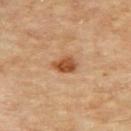Part of a total-body skin-imaging series; this lesion was reviewed on a skin check and was not flagged for biopsy. Automated tile analysis of the lesion measured a lesion area of about 4.5 mm². And it measured a mean CIELAB color near L≈49 a*≈24 b*≈37, a lesion–skin lightness drop of about 14, and a normalized lesion–skin contrast near 10. The software also gave a border-irregularity index near 2/10 and radial color variation of about 1. It also reported a classifier nevus-likeness of about 90/100 and lesion-presence confidence of about 100/100. A 15 mm close-up tile from a total-body photography series done for melanoma screening. The tile uses cross-polarized illumination. The subject is a male about 85 years old or older. Located on the upper back.